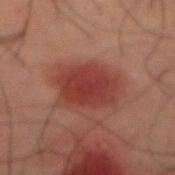Image and clinical context: Automated image analysis of the tile measured an area of roughly 20 mm² and an outline eccentricity of about 0.4 (0 = round, 1 = elongated). And it measured about 8 CIELAB-L* units darker than the surrounding skin and a normalized lesion–skin contrast near 8. The software also gave a classifier nevus-likeness of about 75/100. This is a cross-polarized tile. This image is a 15 mm lesion crop taken from a total-body photograph. A male subject aged approximately 50. On the front of the torso.